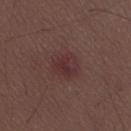The lesion was tiled from a total-body skin photograph and was not biopsied. This is a white-light tile. Located on the lower back. A male subject, in their 30s. A 15 mm crop from a total-body photograph taken for skin-cancer surveillance.